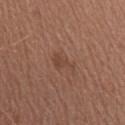{"biopsy_status": "not biopsied; imaged during a skin examination"}